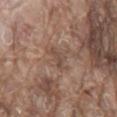{"biopsy_status": "not biopsied; imaged during a skin examination", "lighting": "white-light", "lesion_size": {"long_diameter_mm_approx": 2.5}, "image": {"source": "total-body photography crop", "field_of_view_mm": 15}, "automated_metrics": {"cielab_L": 47, "cielab_a": 17, "cielab_b": 25, "vs_skin_darker_L": 7.0, "vs_skin_contrast_norm": 5.5, "nevus_likeness_0_100": 0, "lesion_detection_confidence_0_100": 95}, "patient": {"sex": "male", "age_approx": 80}, "site": "mid back"}